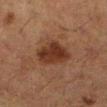Clinical impression:
This lesion was catalogued during total-body skin photography and was not selected for biopsy.